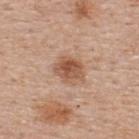- workup: catalogued during a skin exam; not biopsied
- patient: male, aged 53–57
- imaging modality: 15 mm crop, total-body photography
- site: the upper back
- lesion diameter: about 3.5 mm
- automated lesion analysis: an outline eccentricity of about 0.6 (0 = round, 1 = elongated) and two-axis asymmetry of about 0.2; a lesion color around L≈54 a*≈21 b*≈32 in CIELAB, a lesion–skin lightness drop of about 12, and a normalized border contrast of about 8